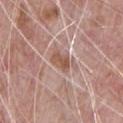Impression:
Part of a total-body skin-imaging series; this lesion was reviewed on a skin check and was not flagged for biopsy.
Image and clinical context:
The tile uses white-light illumination. The total-body-photography lesion software estimated an average lesion color of about L≈54 a*≈20 b*≈27 (CIELAB), a lesion–skin lightness drop of about 10, and a normalized border contrast of about 8. The analysis additionally found border irregularity of about 3 on a 0–10 scale and radial color variation of about 1. Cropped from a whole-body photographic skin survey; the tile spans about 15 mm. Measured at roughly 3 mm in maximum diameter. A male patient, roughly 70 years of age. On the chest.